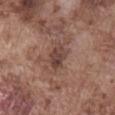Captured during whole-body skin photography for melanoma surveillance; the lesion was not biopsied.
Cropped from a total-body skin-imaging series; the visible field is about 15 mm.
The subject is a male aged 73–77.
The lesion is located on the abdomen.
Longest diameter approximately 3.5 mm.